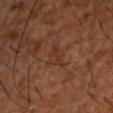{
  "lighting": "cross-polarized",
  "lesion_size": {
    "long_diameter_mm_approx": 4.0
  },
  "site": "chest",
  "patient": {
    "sex": "male",
    "age_approx": 50
  },
  "automated_metrics": {
    "area_mm2_approx": 5.0,
    "eccentricity": 0.85,
    "shape_asymmetry": 0.4,
    "border_irregularity_0_10": 4.5,
    "color_variation_0_10": 1.5,
    "peripheral_color_asymmetry": 0.5
  },
  "image": {
    "source": "total-body photography crop",
    "field_of_view_mm": 15
  }
}